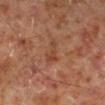Impression:
This lesion was catalogued during total-body skin photography and was not selected for biopsy.
Image and clinical context:
Imaged with cross-polarized lighting. Automated image analysis of the tile measured a footprint of about 4 mm², an outline eccentricity of about 0.8 (0 = round, 1 = elongated), and two-axis asymmetry of about 0.55. The analysis additionally found a mean CIELAB color near L≈40 a*≈22 b*≈30, a lesion–skin lightness drop of about 6, and a normalized border contrast of about 5.5. The software also gave a peripheral color-asymmetry measure near 0. The analysis additionally found a nevus-likeness score of about 0/100 and a detector confidence of about 100 out of 100 that the crop contains a lesion. Longest diameter approximately 3 mm. A male patient roughly 60 years of age. A roughly 15 mm field-of-view crop from a total-body skin photograph. From the right lower leg.